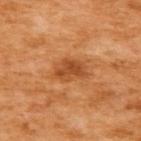Captured during whole-body skin photography for melanoma surveillance; the lesion was not biopsied. Measured at roughly 3.5 mm in maximum diameter. A lesion tile, about 15 mm wide, cut from a 3D total-body photograph. An algorithmic analysis of the crop reported a mean CIELAB color near L≈51 a*≈29 b*≈43, a lesion–skin lightness drop of about 11, and a normalized lesion–skin contrast near 7.5. The analysis additionally found a border-irregularity rating of about 2.5/10, a within-lesion color-variation index near 3.5/10, and radial color variation of about 1.5. The subject is a female aged around 55. On the upper back.